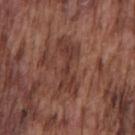Image and clinical context:
Automated tile analysis of the lesion measured a border-irregularity index near 7.5/10 and a within-lesion color-variation index near 3/10. The analysis additionally found a classifier nevus-likeness of about 0/100 and a lesion-detection confidence of about 50/100. A male subject aged 73 to 77. A close-up tile cropped from a whole-body skin photograph, about 15 mm across. This is a white-light tile. Longest diameter approximately 7 mm. The lesion is on the chest.